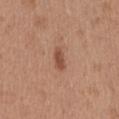Case summary:
• biopsy status: no biopsy performed (imaged during a skin exam)
• subject: female, about 40 years old
• lesion diameter: ~2.5 mm (longest diameter)
• location: the leg
• acquisition: ~15 mm tile from a whole-body skin photo